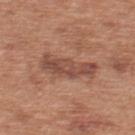Q: Is there a histopathology result?
A: catalogued during a skin exam; not biopsied